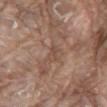Background:
The tile uses white-light illumination. A 15 mm crop from a total-body photograph taken for skin-cancer surveillance. About 2.5 mm across. The total-body-photography lesion software estimated a footprint of about 2.5 mm², an eccentricity of roughly 0.9, and two-axis asymmetry of about 0.3. It also reported a lesion color around L≈48 a*≈18 b*≈27 in CIELAB, about 7 CIELAB-L* units darker than the surrounding skin, and a normalized border contrast of about 5. Located on the mid back. The patient is a male aged approximately 80.